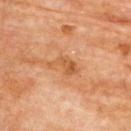Q: Is there a histopathology result?
A: imaged on a skin check; not biopsied
Q: Lesion size?
A: ≈4 mm
Q: What did automated image analysis measure?
A: a footprint of about 5 mm², a shape eccentricity near 0.85, and a shape-asymmetry score of about 0.55 (0 = symmetric); a border-irregularity rating of about 6/10, a color-variation rating of about 3/10, and a peripheral color-asymmetry measure near 1; an automated nevus-likeness rating near 0 out of 100 and lesion-presence confidence of about 100/100
Q: What is the imaging modality?
A: ~15 mm tile from a whole-body skin photo
Q: What are the patient's age and sex?
A: female, aged 78 to 82
Q: Illumination type?
A: cross-polarized illumination
Q: What is the anatomic site?
A: the upper back